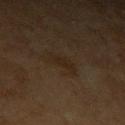<record>
<biopsy_status>not biopsied; imaged during a skin examination</biopsy_status>
<image>
  <source>total-body photography crop</source>
  <field_of_view_mm>15</field_of_view_mm>
</image>
<lighting>cross-polarized</lighting>
<site>left upper arm</site>
<patient>
  <sex>male</sex>
  <age_approx>60</age_approx>
</patient>
<automated_metrics>
  <shape_asymmetry>0.3</shape_asymmetry>
  <cielab_L>20</cielab_L>
  <cielab_a>11</cielab_a>
  <cielab_b>22</cielab_b>
  <vs_skin_darker_L>4.0</vs_skin_darker_L>
  <nevus_likeness_0_100>0</nevus_likeness_0_100>
  <lesion_detection_confidence_0_100>100</lesion_detection_confidence_0_100>
</automated_metrics>
<lesion_size>
  <long_diameter_mm_approx>3.5</long_diameter_mm_approx>
</lesion_size>
</record>